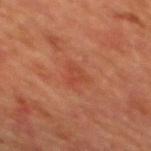biopsy status: imaged on a skin check; not biopsied | location: the mid back | imaging modality: total-body-photography crop, ~15 mm field of view | diameter: about 2.5 mm | subject: male, aged approximately 65 | lighting: cross-polarized.